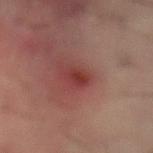Captured during whole-body skin photography for melanoma surveillance; the lesion was not biopsied. A 15 mm close-up extracted from a 3D total-body photography capture. The total-body-photography lesion software estimated an average lesion color of about L≈35 a*≈27 b*≈23 (CIELAB), about 8 CIELAB-L* units darker than the surrounding skin, and a lesion-to-skin contrast of about 7 (normalized; higher = more distinct). Captured under cross-polarized illumination. About 2.5 mm across. From the abdomen. A male patient, aged 58 to 62.